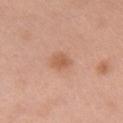The lesion was tiled from a total-body skin photograph and was not biopsied. The lesion is on the chest. Cropped from a total-body skin-imaging series; the visible field is about 15 mm. The patient is a female aged approximately 65. Imaged with white-light lighting. Automated tile analysis of the lesion measured a lesion area of about 4 mm², an outline eccentricity of about 0.65 (0 = round, 1 = elongated), and two-axis asymmetry of about 0.25. The analysis additionally found a color-variation rating of about 2/10 and radial color variation of about 0.5. The analysis additionally found a classifier nevus-likeness of about 75/100 and lesion-presence confidence of about 100/100.